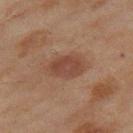The lesion was photographed on a routine skin check and not biopsied; there is no pathology result.
A region of skin cropped from a whole-body photographic capture, roughly 15 mm wide.
Approximately 4 mm at its widest.
From the right thigh.
The subject is a female aged 58–62.
Imaged with cross-polarized lighting.